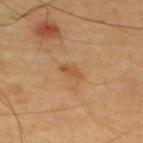<record>
  <biopsy_status>not biopsied; imaged during a skin examination</biopsy_status>
  <lighting>cross-polarized</lighting>
  <site>upper back</site>
  <image>
    <source>total-body photography crop</source>
    <field_of_view_mm>15</field_of_view_mm>
  </image>
  <lesion_size>
    <long_diameter_mm_approx>2.5</long_diameter_mm_approx>
  </lesion_size>
  <patient>
    <sex>male</sex>
    <age_approx>65</age_approx>
  </patient>
</record>